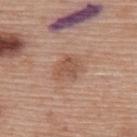Captured during whole-body skin photography for melanoma surveillance; the lesion was not biopsied.
Located on the back.
The lesion's longest dimension is about 2.5 mm.
Cropped from a total-body skin-imaging series; the visible field is about 15 mm.
A female subject, about 60 years old.
Imaged with white-light lighting.